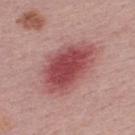biopsy status: catalogued during a skin exam; not biopsied
subject: male, aged 28–32
diameter: ~8 mm (longest diameter)
automated metrics: an average lesion color of about L≈49 a*≈29 b*≈22 (CIELAB), roughly 13 lightness units darker than nearby skin, and a lesion-to-skin contrast of about 9.5 (normalized; higher = more distinct); border irregularity of about 2.5 on a 0–10 scale, internal color variation of about 5.5 on a 0–10 scale, and radial color variation of about 1.5
tile lighting: white-light
imaging modality: total-body-photography crop, ~15 mm field of view